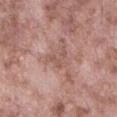Case summary:
- follow-up — catalogued during a skin exam; not biopsied
- subject — male, aged 73–77
- location — the abdomen
- lesion diameter — ≈3 mm
- acquisition — total-body-photography crop, ~15 mm field of view